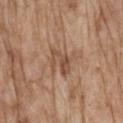The lesion was photographed on a routine skin check and not biopsied; there is no pathology result. About 3.5 mm across. A male patient, aged approximately 70. A lesion tile, about 15 mm wide, cut from a 3D total-body photograph. The lesion is located on the mid back. The tile uses white-light illumination.